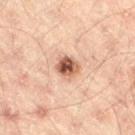Recorded during total-body skin imaging; not selected for excision or biopsy. A male subject aged 43–47. About 3 mm across. The tile uses cross-polarized illumination. The lesion is located on the leg. A 15 mm close-up extracted from a 3D total-body photography capture. The total-body-photography lesion software estimated a lesion color around L≈49 a*≈20 b*≈28 in CIELAB and a normalized lesion–skin contrast near 10.5. The analysis additionally found border irregularity of about 1.5 on a 0–10 scale, a within-lesion color-variation index near 7.5/10, and a peripheral color-asymmetry measure near 2.5.